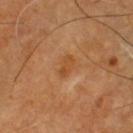Case summary:
* image source: ~15 mm tile from a whole-body skin photo
* illumination: cross-polarized
* anatomic site: the chest
* patient: male, aged 58 to 62